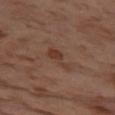Q: Was a biopsy performed?
A: catalogued during a skin exam; not biopsied
Q: What kind of image is this?
A: ~15 mm tile from a whole-body skin photo
Q: Where on the body is the lesion?
A: the left thigh
Q: What lighting was used for the tile?
A: cross-polarized illumination
Q: Who is the patient?
A: female, in their mid-50s
Q: What is the lesion's diameter?
A: ≈3.5 mm
Q: What did automated image analysis measure?
A: a footprint of about 4.5 mm², an outline eccentricity of about 0.9 (0 = round, 1 = elongated), and a shape-asymmetry score of about 0.5 (0 = symmetric); a color-variation rating of about 1/10 and radial color variation of about 0.5